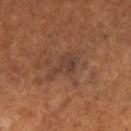Part of a total-body skin-imaging series; this lesion was reviewed on a skin check and was not flagged for biopsy. The lesion-visualizer software estimated two-axis asymmetry of about 0.5. The software also gave a within-lesion color-variation index near 1.5/10 and radial color variation of about 0.5. And it measured an automated nevus-likeness rating near 0 out of 100 and a lesion-detection confidence of about 55/100. Imaged with cross-polarized lighting. The subject is a female aged approximately 80. The lesion is located on the right lower leg. A close-up tile cropped from a whole-body skin photograph, about 15 mm across.